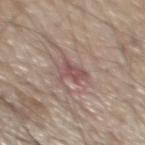follow-up: no biopsy performed (imaged during a skin exam) | image-analysis metrics: border irregularity of about 5.5 on a 0–10 scale; an automated nevus-likeness rating near 0 out of 100 and a detector confidence of about 100 out of 100 that the crop contains a lesion | location: the mid back | imaging modality: 15 mm crop, total-body photography | lesion size: about 3 mm | subject: male, roughly 70 years of age.